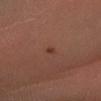Assessment:
Imaged during a routine full-body skin examination; the lesion was not biopsied and no histopathology is available.
Acquisition and patient details:
The lesion is located on the left forearm. A region of skin cropped from a whole-body photographic capture, roughly 15 mm wide. The subject is a male aged around 60.